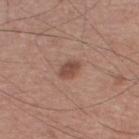Assessment: Imaged during a routine full-body skin examination; the lesion was not biopsied and no histopathology is available. Image and clinical context: The recorded lesion diameter is about 3 mm. From the left lower leg. A 15 mm close-up tile from a total-body photography series done for melanoma screening. The tile uses white-light illumination. Automated image analysis of the tile measured a lesion area of about 4 mm², an outline eccentricity of about 0.75 (0 = round, 1 = elongated), and a shape-asymmetry score of about 0.15 (0 = symmetric). The software also gave a border-irregularity rating of about 1.5/10, a color-variation rating of about 1.5/10, and radial color variation of about 0.5. A male patient about 50 years old.